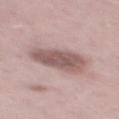No biopsy was performed on this lesion — it was imaged during a full skin examination and was not determined to be concerning. Cropped from a total-body skin-imaging series; the visible field is about 15 mm. The lesion is located on the mid back. Captured under white-light illumination. A male subject roughly 50 years of age. An algorithmic analysis of the crop reported an eccentricity of roughly 0.8.